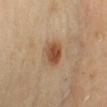workup: catalogued during a skin exam; not biopsied
patient: female, roughly 40 years of age
automated metrics: a lesion color around L≈50 a*≈22 b*≈35 in CIELAB, roughly 12 lightness units darker than nearby skin, and a normalized border contrast of about 9.5; a lesion-detection confidence of about 100/100
illumination: cross-polarized illumination
anatomic site: the chest
image source: 15 mm crop, total-body photography
lesion diameter: ~3 mm (longest diameter)